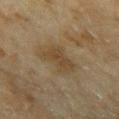The lesion was photographed on a routine skin check and not biopsied; there is no pathology result.
Measured at roughly 3.5 mm in maximum diameter.
The lesion-visualizer software estimated a shape eccentricity near 0.45 and two-axis asymmetry of about 0.3.
A 15 mm close-up tile from a total-body photography series done for melanoma screening.
This is a cross-polarized tile.
A female patient about 60 years old.
The lesion is on the right upper arm.